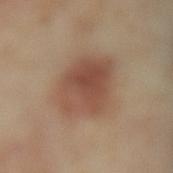No biopsy was performed on this lesion — it was imaged during a full skin examination and was not determined to be concerning. A female patient, aged around 55. From the lower back. This image is a 15 mm lesion crop taken from a total-body photograph.